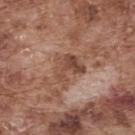The lesion was tiled from a total-body skin photograph and was not biopsied.
A 15 mm crop from a total-body photograph taken for skin-cancer surveillance.
Imaged with white-light lighting.
The lesion is located on the upper back.
The total-body-photography lesion software estimated a mean CIELAB color near L≈47 a*≈21 b*≈28 and roughly 10 lightness units darker than nearby skin.
A male patient roughly 75 years of age.